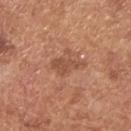Impression:
Captured during whole-body skin photography for melanoma surveillance; the lesion was not biopsied.
Acquisition and patient details:
Captured under white-light illumination. A region of skin cropped from a whole-body photographic capture, roughly 15 mm wide. A male patient, about 65 years old. An algorithmic analysis of the crop reported an average lesion color of about L≈51 a*≈24 b*≈32 (CIELAB) and a normalized border contrast of about 6. And it measured a nevus-likeness score of about 0/100. The recorded lesion diameter is about 4 mm. On the back.